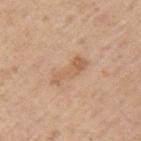notes = no biopsy performed (imaged during a skin exam) | acquisition = ~15 mm crop, total-body skin-cancer survey | TBP lesion metrics = an automated nevus-likeness rating near 0 out of 100 and a lesion-detection confidence of about 100/100 | patient = male, aged approximately 75 | location = the left upper arm | tile lighting = white-light | size = ~5 mm (longest diameter).